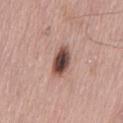Q: Was a biopsy performed?
A: total-body-photography surveillance lesion; no biopsy